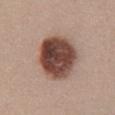{
  "biopsy_status": "not biopsied; imaged during a skin examination",
  "image": {
    "source": "total-body photography crop",
    "field_of_view_mm": 15
  },
  "patient": {
    "sex": "female",
    "age_approx": 25
  },
  "site": "chest",
  "lighting": "white-light",
  "automated_metrics": {
    "cielab_L": 45,
    "cielab_a": 20,
    "cielab_b": 24,
    "vs_skin_darker_L": 20.0,
    "vs_skin_contrast_norm": 13.5,
    "nevus_likeness_0_100": 80,
    "lesion_detection_confidence_0_100": 100
  },
  "lesion_size": {
    "long_diameter_mm_approx": 6.0
  }
}